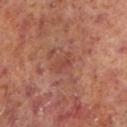This lesion was catalogued during total-body skin photography and was not selected for biopsy. A region of skin cropped from a whole-body photographic capture, roughly 15 mm wide. A male patient, aged 63 to 67. Automated image analysis of the tile measured a lesion color around L≈44 a*≈26 b*≈28 in CIELAB and a normalized border contrast of about 5. The software also gave a nevus-likeness score of about 0/100. Located on the left lower leg. This is a cross-polarized tile. About 2.5 mm across.